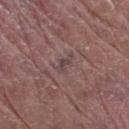{"biopsy_status": "not biopsied; imaged during a skin examination", "automated_metrics": {"area_mm2_approx": 2.5, "eccentricity": 0.9, "shape_asymmetry": 0.45, "vs_skin_darker_L": 7.0, "vs_skin_contrast_norm": 6.0, "border_irregularity_0_10": 5.0, "color_variation_0_10": 0.0, "peripheral_color_asymmetry": 0.0}, "lesion_size": {"long_diameter_mm_approx": 2.5}, "patient": {"sex": "male", "age_approx": 80}, "lighting": "white-light", "image": {"source": "total-body photography crop", "field_of_view_mm": 15}, "site": "leg"}